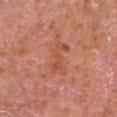Assessment: The lesion was photographed on a routine skin check and not biopsied; there is no pathology result. Context: Approximately 4 mm at its widest. Located on the chest. A male patient roughly 65 years of age. The tile uses white-light illumination. A close-up tile cropped from a whole-body skin photograph, about 15 mm across.